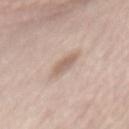location = the mid back | image = ~15 mm crop, total-body skin-cancer survey | subject = female, aged 63 to 67 | diameter = ~4 mm (longest diameter) | illumination = white-light.